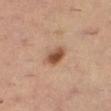A roughly 15 mm field-of-view crop from a total-body skin photograph. An algorithmic analysis of the crop reported internal color variation of about 5 on a 0–10 scale. It also reported a lesion-detection confidence of about 100/100. This is a cross-polarized tile. Located on the leg. The subject is a female aged approximately 35.